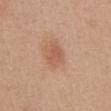Part of a total-body skin-imaging series; this lesion was reviewed on a skin check and was not flagged for biopsy.